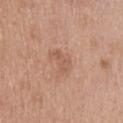Notes:
• image source — total-body-photography crop, ~15 mm field of view
• size — about 3 mm
• site — the upper back
• patient — male, aged 38–42
• lighting — white-light illumination